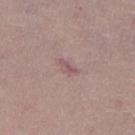follow-up: no biopsy performed (imaged during a skin exam)
patient: male, aged 43–47
site: the right lower leg
size: ≈2.5 mm
image: ~15 mm crop, total-body skin-cancer survey
illumination: white-light